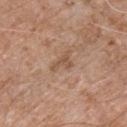- workup — imaged on a skin check; not biopsied
- lighting — white-light
- subject — male, approximately 55 years of age
- image-analysis metrics — an area of roughly 3 mm², an eccentricity of roughly 0.8, and a shape-asymmetry score of about 0.6 (0 = symmetric); a within-lesion color-variation index near 0.5/10
- acquisition — ~15 mm tile from a whole-body skin photo
- anatomic site — the chest
- lesion size — ~2.5 mm (longest diameter)